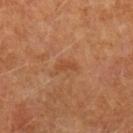{
  "biopsy_status": "not biopsied; imaged during a skin examination",
  "lighting": "cross-polarized",
  "image": {
    "source": "total-body photography crop",
    "field_of_view_mm": 15
  },
  "site": "arm",
  "patient": {
    "sex": "male",
    "age_approx": 55
  },
  "lesion_size": {
    "long_diameter_mm_approx": 2.5
  },
  "automated_metrics": {
    "border_irregularity_0_10": 4.0,
    "color_variation_0_10": 0.0,
    "peripheral_color_asymmetry": 0.0
  }
}